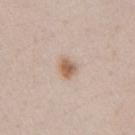Part of a total-body skin-imaging series; this lesion was reviewed on a skin check and was not flagged for biopsy.
Located on the right upper arm.
A female subject, in their 40s.
A roughly 15 mm field-of-view crop from a total-body skin photograph.
Measured at roughly 2.5 mm in maximum diameter.
Captured under white-light illumination.
An algorithmic analysis of the crop reported an area of roughly 4.5 mm², a shape eccentricity near 0.65, and a shape-asymmetry score of about 0.3 (0 = symmetric). The analysis additionally found a lesion color around L≈61 a*≈16 b*≈29 in CIELAB, roughly 11 lightness units darker than nearby skin, and a normalized lesion–skin contrast near 8.